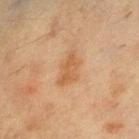  biopsy_status: not biopsied; imaged during a skin examination
  patient:
    sex: female
    age_approx: 55
  image:
    source: total-body photography crop
    field_of_view_mm: 15
  site: left lower leg